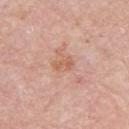Impression:
The lesion was tiled from a total-body skin photograph and was not biopsied.
Background:
The lesion's longest dimension is about 2.5 mm. Located on the left upper arm. The tile uses white-light illumination. A lesion tile, about 15 mm wide, cut from a 3D total-body photograph. A female subject, aged 63 to 67.